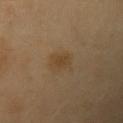| field | value |
|---|---|
| follow-up | no biopsy performed (imaged during a skin exam) |
| image-analysis metrics | an outline eccentricity of about 0.8 (0 = round, 1 = elongated) and two-axis asymmetry of about 0.15; a lesion–skin lightness drop of about 6 and a normalized border contrast of about 6; border irregularity of about 1.5 on a 0–10 scale, a within-lesion color-variation index near 1.5/10, and peripheral color asymmetry of about 0.5 |
| image | ~15 mm tile from a whole-body skin photo |
| lesion diameter | ≈3 mm |
| body site | the left upper arm |
| patient | male, roughly 40 years of age |
| illumination | cross-polarized illumination |